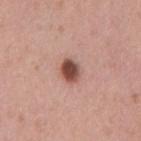No biopsy was performed on this lesion — it was imaged during a full skin examination and was not determined to be concerning. On the mid back. Imaged with white-light lighting. Cropped from a whole-body photographic skin survey; the tile spans about 15 mm. A male patient, approximately 40 years of age.